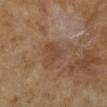follow-up: no biopsy performed (imaged during a skin exam); size: ≈3 mm; acquisition: ~15 mm crop, total-body skin-cancer survey; site: the leg; illumination: cross-polarized illumination; patient: male, aged 63–67.